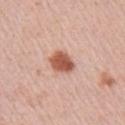| key | value |
|---|---|
| follow-up | no biopsy performed (imaged during a skin exam) |
| automated lesion analysis | a shape eccentricity near 0.65 and a shape-asymmetry score of about 0.2 (0 = symmetric); a lesion color around L≈56 a*≈26 b*≈31 in CIELAB, roughly 16 lightness units darker than nearby skin, and a normalized lesion–skin contrast near 10.5; border irregularity of about 2 on a 0–10 scale, a within-lesion color-variation index near 3/10, and peripheral color asymmetry of about 1 |
| site | the right upper arm |
| patient | female, aged around 60 |
| image source | total-body-photography crop, ~15 mm field of view |